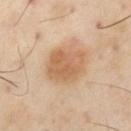Assessment:
The lesion was photographed on a routine skin check and not biopsied; there is no pathology result.
Clinical summary:
Located on the left thigh. The patient is a male about 55 years old. A close-up tile cropped from a whole-body skin photograph, about 15 mm across.